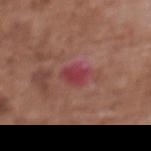Part of a total-body skin-imaging series; this lesion was reviewed on a skin check and was not flagged for biopsy. The lesion is located on the upper back. The tile uses white-light illumination. Cropped from a whole-body photographic skin survey; the tile spans about 15 mm. Measured at roughly 4 mm in maximum diameter. A female patient in their mid-70s.